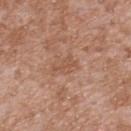Clinical impression: Recorded during total-body skin imaging; not selected for excision or biopsy. Acquisition and patient details: A male subject, aged approximately 45. A 15 mm crop from a total-body photograph taken for skin-cancer surveillance. From the upper back.